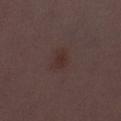The patient is a female approximately 30 years of age. A 15 mm close-up extracted from a 3D total-body photography capture. Located on the right thigh.